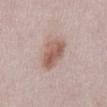– biopsy status — catalogued during a skin exam; not biopsied
– body site — the abdomen
– lesion size — ~4 mm (longest diameter)
– patient — female, in their 50s
– tile lighting — white-light
– image — ~15 mm crop, total-body skin-cancer survey